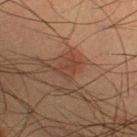Part of a total-body skin-imaging series; this lesion was reviewed on a skin check and was not flagged for biopsy. Captured under cross-polarized illumination. Longest diameter approximately 2.5 mm. A male subject approximately 35 years of age. Located on the right lower leg. A 15 mm close-up tile from a total-body photography series done for melanoma screening.